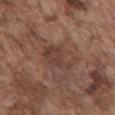Q: Who is the patient?
A: male, in their mid-70s
Q: Lesion size?
A: ~4.5 mm (longest diameter)
Q: What is the anatomic site?
A: the front of the torso
Q: How was this image acquired?
A: ~15 mm tile from a whole-body skin photo